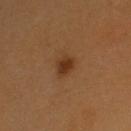The lesion was tiled from a total-body skin photograph and was not biopsied.
A 15 mm close-up tile from a total-body photography series done for melanoma screening.
The lesion's longest dimension is about 2.5 mm.
The total-body-photography lesion software estimated a lesion color around L≈35 a*≈20 b*≈33 in CIELAB, roughly 9 lightness units darker than nearby skin, and a lesion-to-skin contrast of about 8 (normalized; higher = more distinct). It also reported a classifier nevus-likeness of about 95/100 and a detector confidence of about 100 out of 100 that the crop contains a lesion.
A male patient, aged 58–62.
On the head or neck.